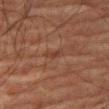The lesion was tiled from a total-body skin photograph and was not biopsied. Approximately 2.5 mm at its widest. The subject is a male in their 80s. This is a cross-polarized tile. The lesion is on the right thigh. The total-body-photography lesion software estimated a mean CIELAB color near L≈30 a*≈18 b*≈23, about 5 CIELAB-L* units darker than the surrounding skin, and a normalized lesion–skin contrast near 5. The software also gave a border-irregularity rating of about 3.5/10. The software also gave an automated nevus-likeness rating near 0 out of 100 and lesion-presence confidence of about 100/100. A close-up tile cropped from a whole-body skin photograph, about 15 mm across.